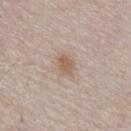  biopsy_status: not biopsied; imaged during a skin examination
  image:
    source: total-body photography crop
    field_of_view_mm: 15
  patient:
    sex: male
    age_approx: 65
  site: chest
  lighting: white-light
  lesion_size:
    long_diameter_mm_approx: 3.0